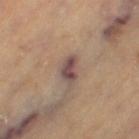This lesion was catalogued during total-body skin photography and was not selected for biopsy. A female subject, in their 70s. The recorded lesion diameter is about 3 mm. The lesion is located on the left thigh. A lesion tile, about 15 mm wide, cut from a 3D total-body photograph. The lesion-visualizer software estimated an area of roughly 4.5 mm² and a symmetry-axis asymmetry near 0.35. It also reported a lesion color around L≈47 a*≈17 b*≈20 in CIELAB, roughly 13 lightness units darker than nearby skin, and a lesion-to-skin contrast of about 11 (normalized; higher = more distinct). And it measured a border-irregularity rating of about 3.5/10, a within-lesion color-variation index near 2/10, and a peripheral color-asymmetry measure near 0.5. Captured under cross-polarized illumination.